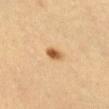Q: Is there a histopathology result?
A: catalogued during a skin exam; not biopsied
Q: What is the anatomic site?
A: the abdomen
Q: Patient demographics?
A: female, about 50 years old
Q: What is the imaging modality?
A: total-body-photography crop, ~15 mm field of view